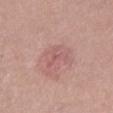Impression: This lesion was catalogued during total-body skin photography and was not selected for biopsy. Acquisition and patient details: From the left thigh. A region of skin cropped from a whole-body photographic capture, roughly 15 mm wide. This is a white-light tile. A female subject about 40 years old.